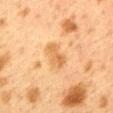Imaged during a routine full-body skin examination; the lesion was not biopsied and no histopathology is available.
A 15 mm close-up extracted from a 3D total-body photography capture.
A female subject, aged around 40.
The lesion is located on the back.